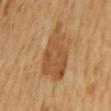Captured during whole-body skin photography for melanoma surveillance; the lesion was not biopsied. This is a cross-polarized tile. The lesion is on the mid back. Cropped from a whole-body photographic skin survey; the tile spans about 15 mm. A female subject about 55 years old.